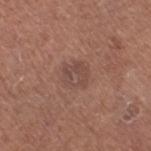The lesion was photographed on a routine skin check and not biopsied; there is no pathology result. Located on the right thigh. Approximately 3 mm at its widest. Automated image analysis of the tile measured a footprint of about 7 mm², an eccentricity of roughly 0.4, and two-axis asymmetry of about 0.25. The analysis additionally found about 6 CIELAB-L* units darker than the surrounding skin. And it measured border irregularity of about 2.5 on a 0–10 scale, internal color variation of about 4 on a 0–10 scale, and peripheral color asymmetry of about 1.5. The software also gave an automated nevus-likeness rating near 0 out of 100 and lesion-presence confidence of about 100/100. A female patient approximately 50 years of age. This image is a 15 mm lesion crop taken from a total-body photograph.